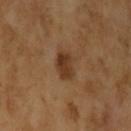The lesion was tiled from a total-body skin photograph and was not biopsied. Imaged with cross-polarized lighting. Automated image analysis of the tile measured an average lesion color of about L≈37 a*≈20 b*≈33 (CIELAB), roughly 10 lightness units darker than nearby skin, and a normalized lesion–skin contrast near 9. It also reported a peripheral color-asymmetry measure near 1.5. The analysis additionally found a classifier nevus-likeness of about 80/100. A region of skin cropped from a whole-body photographic capture, roughly 15 mm wide. The lesion is located on the left upper arm. A female subject, approximately 70 years of age. The lesion's longest dimension is about 3 mm.